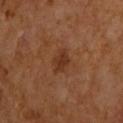Part of a total-body skin-imaging series; this lesion was reviewed on a skin check and was not flagged for biopsy.
The lesion is on the left upper arm.
The tile uses cross-polarized illumination.
A region of skin cropped from a whole-body photographic capture, roughly 15 mm wide.
The subject is a male roughly 65 years of age.
The total-body-photography lesion software estimated an area of roughly 5 mm², an outline eccentricity of about 0.65 (0 = round, 1 = elongated), and a symmetry-axis asymmetry near 0.2. And it measured roughly 7 lightness units darker than nearby skin and a normalized lesion–skin contrast near 6.5. The analysis additionally found internal color variation of about 1.5 on a 0–10 scale and radial color variation of about 0.5. It also reported a classifier nevus-likeness of about 30/100 and lesion-presence confidence of about 100/100.